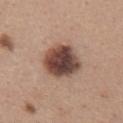workup: catalogued during a skin exam; not biopsied | subject: female, roughly 40 years of age | location: the upper back | tile lighting: white-light illumination | image: 15 mm crop, total-body photography | lesion size: ≈4.5 mm.